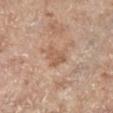No biopsy was performed on this lesion — it was imaged during a full skin examination and was not determined to be concerning. From the left lower leg. A female patient, aged 83 or over. Approximately 3 mm at its widest. A 15 mm crop from a total-body photograph taken for skin-cancer surveillance.